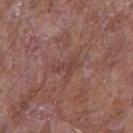size: about 3 mm; patient: male, aged 63 to 67; tile lighting: white-light; anatomic site: the right upper arm; acquisition: ~15 mm tile from a whole-body skin photo.